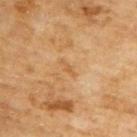Clinical impression:
Captured during whole-body skin photography for melanoma surveillance; the lesion was not biopsied.
Background:
A male subject, aged around 65. On the back. A close-up tile cropped from a whole-body skin photograph, about 15 mm across. This is a cross-polarized tile. The total-body-photography lesion software estimated border irregularity of about 3.5 on a 0–10 scale, internal color variation of about 0 on a 0–10 scale, and radial color variation of about 0.